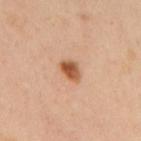Clinical impression:
No biopsy was performed on this lesion — it was imaged during a full skin examination and was not determined to be concerning.
Image and clinical context:
The lesion is on the upper back. The lesion's longest dimension is about 3 mm. Captured under cross-polarized illumination. A roughly 15 mm field-of-view crop from a total-body skin photograph. A female subject approximately 40 years of age. The lesion-visualizer software estimated a footprint of about 4.5 mm², an eccentricity of roughly 0.75, and a shape-asymmetry score of about 0.2 (0 = symmetric). It also reported a lesion color around L≈55 a*≈24 b*≈36 in CIELAB, a lesion–skin lightness drop of about 15, and a lesion-to-skin contrast of about 10 (normalized; higher = more distinct). The analysis additionally found internal color variation of about 5 on a 0–10 scale and peripheral color asymmetry of about 2.